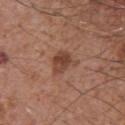Notes:
• biopsy status · catalogued during a skin exam; not biopsied
• body site · the front of the torso
• patient · male, in their mid- to late 60s
• image source · 15 mm crop, total-body photography